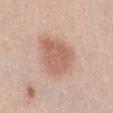  biopsy_status: not biopsied; imaged during a skin examination
  site: abdomen
  patient:
    sex: female
    age_approx: 60
  image:
    source: total-body photography crop
    field_of_view_mm: 15